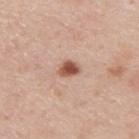| field | value |
|---|---|
| follow-up | total-body-photography surveillance lesion; no biopsy |
| image source | 15 mm crop, total-body photography |
| location | the upper back |
| subject | male, about 45 years old |